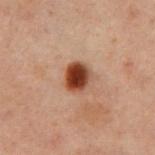Q: Is there a histopathology result?
A: no biopsy performed (imaged during a skin exam)
Q: What lighting was used for the tile?
A: cross-polarized
Q: Patient demographics?
A: male, aged approximately 60
Q: Lesion size?
A: ~3 mm (longest diameter)
Q: Lesion location?
A: the front of the torso
Q: How was this image acquired?
A: ~15 mm crop, total-body skin-cancer survey